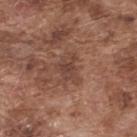This lesion was catalogued during total-body skin photography and was not selected for biopsy. A 15 mm close-up tile from a total-body photography series done for melanoma screening. The subject is a male in their mid-70s. The tile uses white-light illumination. The total-body-photography lesion software estimated a footprint of about 7 mm² and two-axis asymmetry of about 0.5. The analysis additionally found a normalized border contrast of about 5.5. And it measured a border-irregularity rating of about 5/10. The lesion's longest dimension is about 3.5 mm. The lesion is on the back.